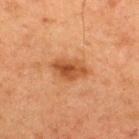Captured during whole-body skin photography for melanoma surveillance; the lesion was not biopsied. A male subject aged approximately 60. Measured at roughly 4.5 mm in maximum diameter. This is a cross-polarized tile. A 15 mm crop from a total-body photograph taken for skin-cancer surveillance. From the upper back.